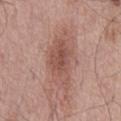Clinical impression:
Part of a total-body skin-imaging series; this lesion was reviewed on a skin check and was not flagged for biopsy.